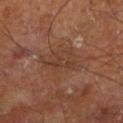Clinical impression: The lesion was tiled from a total-body skin photograph and was not biopsied. Context: Captured under cross-polarized illumination. Longest diameter approximately 5 mm. Located on the right leg. The subject is a male approximately 60 years of age. Cropped from a whole-body photographic skin survey; the tile spans about 15 mm.